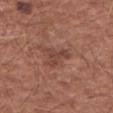Captured during whole-body skin photography for melanoma surveillance; the lesion was not biopsied. This is a white-light tile. Longest diameter approximately 4 mm. A 15 mm crop from a total-body photograph taken for skin-cancer surveillance. On the left forearm. A male patient about 65 years old.